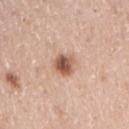subject=female, aged 48 to 52 | location=the right upper arm | lighting=white-light illumination | image=15 mm crop, total-body photography | lesion diameter=≈2.5 mm.